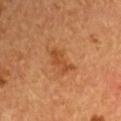Clinical impression: Part of a total-body skin-imaging series; this lesion was reviewed on a skin check and was not flagged for biopsy. Background: A male patient, aged around 55. About 3.5 mm across. A 15 mm close-up extracted from a 3D total-body photography capture. From the left upper arm. Imaged with cross-polarized lighting.